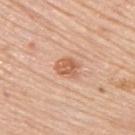Clinical impression: This lesion was catalogued during total-body skin photography and was not selected for biopsy. Image and clinical context: A male subject, about 80 years old. The tile uses white-light illumination. Cropped from a whole-body photographic skin survey; the tile spans about 15 mm. The lesion is located on the back.